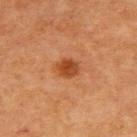Clinical impression: No biopsy was performed on this lesion — it was imaged during a full skin examination and was not determined to be concerning.